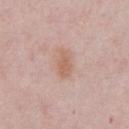Impression:
This lesion was catalogued during total-body skin photography and was not selected for biopsy.
Clinical summary:
The lesion is located on the chest. The total-body-photography lesion software estimated a lesion color around L≈62 a*≈20 b*≈28 in CIELAB, about 8 CIELAB-L* units darker than the surrounding skin, and a lesion-to-skin contrast of about 6 (normalized; higher = more distinct). It also reported a border-irregularity rating of about 2/10, a color-variation rating of about 2/10, and radial color variation of about 0.5. This is a white-light tile. A 15 mm crop from a total-body photograph taken for skin-cancer surveillance. About 3.5 mm across. A male patient, in their mid- to late 60s.